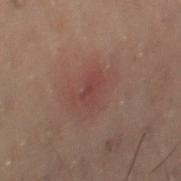Case summary:
* notes: no biopsy performed (imaged during a skin exam)
* anatomic site: the left leg
* subject: female, aged 53–57
* illumination: cross-polarized illumination
* image source: total-body-photography crop, ~15 mm field of view